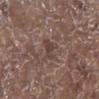- follow-up — no biopsy performed (imaged during a skin exam)
- lesion size — about 2.5 mm
- patient — male, aged around 70
- anatomic site — the right lower leg
- image source — ~15 mm tile from a whole-body skin photo